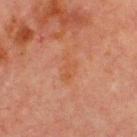biopsy status: total-body-photography surveillance lesion; no biopsy
subject: male, aged 68–72
location: the chest
image source: ~15 mm tile from a whole-body skin photo